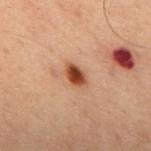– biopsy status · no biopsy performed (imaged during a skin exam)
– lighting · cross-polarized
– diameter · about 3 mm
– automated lesion analysis · about 13 CIELAB-L* units darker than the surrounding skin and a lesion-to-skin contrast of about 11.5 (normalized; higher = more distinct); a border-irregularity rating of about 2/10, a color-variation rating of about 4/10, and peripheral color asymmetry of about 1
– patient · male, in their 60s
– imaging modality · total-body-photography crop, ~15 mm field of view
– site · the back